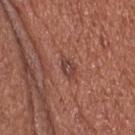  biopsy_status: not biopsied; imaged during a skin examination
  lesion_size:
    long_diameter_mm_approx: 2.5
  lighting: white-light
  automated_metrics:
    area_mm2_approx: 3.0
    eccentricity: 0.85
    shape_asymmetry: 0.35
    nevus_likeness_0_100: 0
    lesion_detection_confidence_0_100: 100
  patient:
    sex: male
    age_approx: 55
  site: head or neck
  image:
    source: total-body photography crop
    field_of_view_mm: 15